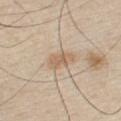Part of a total-body skin-imaging series; this lesion was reviewed on a skin check and was not flagged for biopsy. The tile uses white-light illumination. The total-body-photography lesion software estimated an outline eccentricity of about 0.8 (0 = round, 1 = elongated) and a symmetry-axis asymmetry near 0.2. The software also gave a lesion color around L≈62 a*≈15 b*≈32 in CIELAB and a lesion–skin lightness drop of about 9. The software also gave a border-irregularity rating of about 2.5/10, a color-variation rating of about 2.5/10, and radial color variation of about 1. It also reported a classifier nevus-likeness of about 50/100 and a lesion-detection confidence of about 100/100. Approximately 3 mm at its widest. A 15 mm close-up tile from a total-body photography series done for melanoma screening. A male subject, aged approximately 30. Located on the chest.